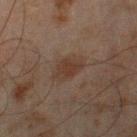Part of a total-body skin-imaging series; this lesion was reviewed on a skin check and was not flagged for biopsy. Captured under cross-polarized illumination. A lesion tile, about 15 mm wide, cut from a 3D total-body photograph. A male patient, aged approximately 45. The total-body-photography lesion software estimated an area of roughly 8 mm² and an eccentricity of roughly 0.7. It also reported a border-irregularity rating of about 2/10 and radial color variation of about 1. The software also gave a lesion-detection confidence of about 100/100. The lesion is on the right thigh. The recorded lesion diameter is about 3.5 mm.